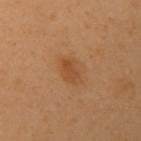The lesion was photographed on a routine skin check and not biopsied; there is no pathology result. A region of skin cropped from a whole-body photographic capture, roughly 15 mm wide. Captured under cross-polarized illumination. On the chest. The subject is a female aged around 60. About 3 mm across.